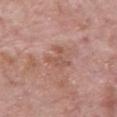The lesion was photographed on a routine skin check and not biopsied; there is no pathology result. A male patient aged around 80. The lesion is located on the chest. Longest diameter approximately 3 mm. A 15 mm close-up tile from a total-body photography series done for melanoma screening.